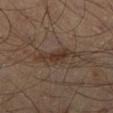Impression: No biopsy was performed on this lesion — it was imaged during a full skin examination and was not determined to be concerning. Clinical summary: This image is a 15 mm lesion crop taken from a total-body photograph. The lesion's longest dimension is about 5.5 mm. On the right lower leg. The subject is a male in their mid- to late 50s.